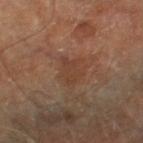This lesion was catalogued during total-body skin photography and was not selected for biopsy. Imaged with cross-polarized lighting. A lesion tile, about 15 mm wide, cut from a 3D total-body photograph. Longest diameter approximately 3.5 mm. The patient is a male aged around 70. Automated image analysis of the tile measured a lesion color around L≈39 a*≈19 b*≈28 in CIELAB and a normalized border contrast of about 5. The software also gave an automated nevus-likeness rating near 0 out of 100 and a detector confidence of about 100 out of 100 that the crop contains a lesion. Located on the right thigh.